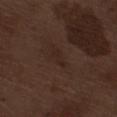The lesion is located on the right thigh. Imaged with white-light lighting. A male subject, aged around 70. Approximately 2.5 mm at its widest. A 15 mm close-up tile from a total-body photography series done for melanoma screening.